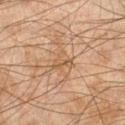Assessment:
The lesion was tiled from a total-body skin photograph and was not biopsied.
Acquisition and patient details:
The subject is a male approximately 45 years of age. About 3 mm across. This is a cross-polarized tile. On the left lower leg. Cropped from a whole-body photographic skin survey; the tile spans about 15 mm.